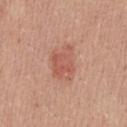{"biopsy_status": "not biopsied; imaged during a skin examination", "image": {"source": "total-body photography crop", "field_of_view_mm": 15}, "lesion_size": {"long_diameter_mm_approx": 4.5}, "automated_metrics": {"area_mm2_approx": 10.0, "eccentricity": 0.75, "shape_asymmetry": 0.25, "nevus_likeness_0_100": 65}, "site": "front of the torso", "patient": {"sex": "male", "age_approx": 25}}